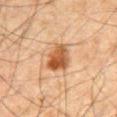Impression:
No biopsy was performed on this lesion — it was imaged during a full skin examination and was not determined to be concerning.
Context:
A male patient approximately 65 years of age. The lesion's longest dimension is about 3.5 mm. Automated tile analysis of the lesion measured an area of roughly 9 mm². The analysis additionally found an average lesion color of about L≈49 a*≈21 b*≈35 (CIELAB), about 13 CIELAB-L* units darker than the surrounding skin, and a normalized lesion–skin contrast near 10. It also reported border irregularity of about 2 on a 0–10 scale, a color-variation rating of about 6.5/10, and peripheral color asymmetry of about 2. A 15 mm close-up tile from a total-body photography series done for melanoma screening. Located on the abdomen. Imaged with cross-polarized lighting.